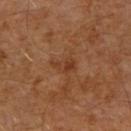follow-up: total-body-photography surveillance lesion; no biopsy
patient: male, approximately 60 years of age
automated metrics: a lesion area of about 4 mm², an outline eccentricity of about 0.8 (0 = round, 1 = elongated), and a shape-asymmetry score of about 0.4 (0 = symmetric); a mean CIELAB color near L≈37 a*≈23 b*≈32 and a lesion-to-skin contrast of about 6 (normalized; higher = more distinct); a classifier nevus-likeness of about 0/100
site: the right upper arm
acquisition: ~15 mm crop, total-body skin-cancer survey
lesion diameter: ≈3 mm